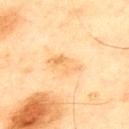A region of skin cropped from a whole-body photographic capture, roughly 15 mm wide. Longest diameter approximately 4 mm. The tile uses cross-polarized illumination. From the back. A male patient in their mid-40s.